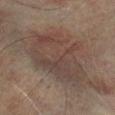Case summary:
* workup: no biopsy performed (imaged during a skin exam)
* lesion diameter: ~8 mm (longest diameter)
* lighting: cross-polarized
* body site: the right lower leg
* image: 15 mm crop, total-body photography
* patient: male, aged approximately 75
* TBP lesion metrics: an average lesion color of about L≈35 a*≈12 b*≈18 (CIELAB), a lesion–skin lightness drop of about 6, and a normalized border contrast of about 6